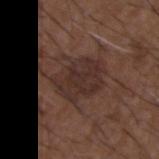| key | value |
|---|---|
| follow-up | imaged on a skin check; not biopsied |
| anatomic site | the back |
| image source | ~15 mm tile from a whole-body skin photo |
| tile lighting | white-light |
| patient | male, aged around 50 |
| size | ~5.5 mm (longest diameter) |